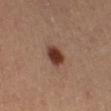Recorded during total-body skin imaging; not selected for excision or biopsy. About 3 mm across. A lesion tile, about 15 mm wide, cut from a 3D total-body photograph. A female patient in their mid-40s. From the leg.